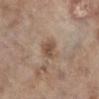The lesion was photographed on a routine skin check and not biopsied; there is no pathology result.
About 3 mm across.
A close-up tile cropped from a whole-body skin photograph, about 15 mm across.
A female subject about 85 years old or older.
Imaged with white-light lighting.
The lesion is on the right lower leg.